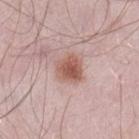Notes:
• location — the left thigh
• patient — male, aged around 55
• lesion size — about 3.5 mm
• image — 15 mm crop, total-body photography
• image-analysis metrics — a classifier nevus-likeness of about 95/100